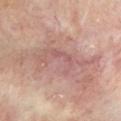notes: catalogued during a skin exam; not biopsied
subject: female, aged around 60
acquisition: total-body-photography crop, ~15 mm field of view
diameter: ~8 mm (longest diameter)
anatomic site: the chest
automated metrics: a border-irregularity rating of about 8.5/10, internal color variation of about 4 on a 0–10 scale, and radial color variation of about 1
lighting: cross-polarized